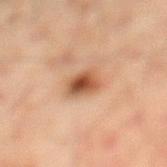The lesion was tiled from a total-body skin photograph and was not biopsied. Automated image analysis of the tile measured an outline eccentricity of about 0.75 (0 = round, 1 = elongated) and a symmetry-axis asymmetry near 0.25. It also reported a lesion color around L≈47 a*≈20 b*≈31 in CIELAB, a lesion–skin lightness drop of about 13, and a normalized border contrast of about 9.5. The lesion is located on the leg. This is a cross-polarized tile. About 3.5 mm across. A close-up tile cropped from a whole-body skin photograph, about 15 mm across. A male subject aged approximately 45.